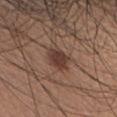Clinical impression:
Recorded during total-body skin imaging; not selected for excision or biopsy.
Acquisition and patient details:
The lesion is located on the head or neck. Longest diameter approximately 3.5 mm. An algorithmic analysis of the crop reported an area of roughly 7 mm², an outline eccentricity of about 0.7 (0 = round, 1 = elongated), and a symmetry-axis asymmetry near 0.2. The analysis additionally found a mean CIELAB color near L≈39 a*≈18 b*≈24, roughly 10 lightness units darker than nearby skin, and a normalized border contrast of about 8.5. And it measured a within-lesion color-variation index near 3/10 and radial color variation of about 1. The analysis additionally found a nevus-likeness score of about 85/100 and a detector confidence of about 100 out of 100 that the crop contains a lesion. A female patient roughly 55 years of age. This is a white-light tile. A lesion tile, about 15 mm wide, cut from a 3D total-body photograph.